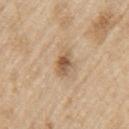The lesion was photographed on a routine skin check and not biopsied; there is no pathology result.